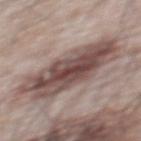Impression: The lesion was tiled from a total-body skin photograph and was not biopsied. Background: The lesion is on the mid back. The recorded lesion diameter is about 9.5 mm. Cropped from a total-body skin-imaging series; the visible field is about 15 mm. The subject is a male aged 63 to 67.